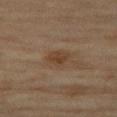Assessment:
This lesion was catalogued during total-body skin photography and was not selected for biopsy.
Context:
The lesion is located on the right thigh. A 15 mm crop from a total-body photograph taken for skin-cancer surveillance. The patient is a female aged around 60.